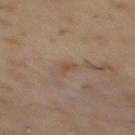<lesion>
  <lesion_size>
    <long_diameter_mm_approx>1.5</long_diameter_mm_approx>
  </lesion_size>
  <site>mid back</site>
  <patient>
    <sex>male</sex>
    <age_approx>55</age_approx>
  </patient>
  <image>
    <source>total-body photography crop</source>
    <field_of_view_mm>15</field_of_view_mm>
  </image>
  <lighting>cross-polarized</lighting>
</lesion>